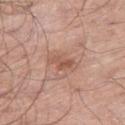follow-up = total-body-photography surveillance lesion; no biopsy
lesion diameter = about 3.5 mm
body site = the right thigh
imaging modality = ~15 mm tile from a whole-body skin photo
TBP lesion metrics = an average lesion color of about L≈55 a*≈22 b*≈29 (CIELAB), roughly 9 lightness units darker than nearby skin, and a normalized border contrast of about 6.5; a classifier nevus-likeness of about 25/100 and a lesion-detection confidence of about 100/100
patient = male, about 70 years old
lighting = white-light illumination